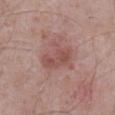| key | value |
|---|---|
| biopsy status | total-body-photography surveillance lesion; no biopsy |
| acquisition | 15 mm crop, total-body photography |
| size | ≈4.5 mm |
| patient | male, approximately 70 years of age |
| image-analysis metrics | an average lesion color of about L≈50 a*≈23 b*≈23 (CIELAB), roughly 8 lightness units darker than nearby skin, and a lesion-to-skin contrast of about 6 (normalized; higher = more distinct); a color-variation rating of about 3/10 and radial color variation of about 1; an automated nevus-likeness rating near 5 out of 100 and a lesion-detection confidence of about 100/100 |
| anatomic site | the abdomen |
| tile lighting | white-light |